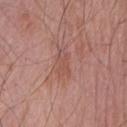Q: Was a biopsy performed?
A: total-body-photography surveillance lesion; no biopsy
Q: What kind of image is this?
A: ~15 mm crop, total-body skin-cancer survey
Q: How large is the lesion?
A: about 3.5 mm
Q: Lesion location?
A: the front of the torso
Q: Patient demographics?
A: male, approximately 75 years of age